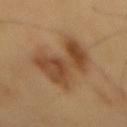No biopsy was performed on this lesion — it was imaged during a full skin examination and was not determined to be concerning.
The lesion is on the mid back.
Longest diameter approximately 8 mm.
The total-body-photography lesion software estimated a footprint of about 25 mm², a shape eccentricity near 0.85, and a shape-asymmetry score of about 0.5 (0 = symmetric). It also reported a mean CIELAB color near L≈45 a*≈19 b*≈33, about 10 CIELAB-L* units darker than the surrounding skin, and a lesion-to-skin contrast of about 8 (normalized; higher = more distinct).
A 15 mm close-up extracted from a 3D total-body photography capture.
Imaged with cross-polarized lighting.
A male patient, aged 58 to 62.